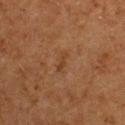Q: Where on the body is the lesion?
A: the upper back
Q: How large is the lesion?
A: ~2.5 mm (longest diameter)
Q: What did automated image analysis measure?
A: a footprint of about 2.5 mm², an eccentricity of roughly 0.95, and a shape-asymmetry score of about 0.25 (0 = symmetric); a border-irregularity rating of about 3/10, a color-variation rating of about 0/10, and radial color variation of about 0
Q: What kind of image is this?
A: ~15 mm tile from a whole-body skin photo
Q: Patient demographics?
A: female, approximately 50 years of age
Q: How was the tile lit?
A: cross-polarized